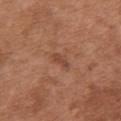The lesion was photographed on a routine skin check and not biopsied; there is no pathology result. A 15 mm close-up tile from a total-body photography series done for melanoma screening. A female subject, approximately 65 years of age. The lesion is on the chest.